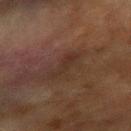Recorded during total-body skin imaging; not selected for excision or biopsy.
The lesion is located on the right forearm.
Approximately 4 mm at its widest.
The patient is a male aged approximately 70.
Automated image analysis of the tile measured a lesion area of about 5 mm². The software also gave a mean CIELAB color near L≈25 a*≈14 b*≈21 and a lesion-to-skin contrast of about 5.5 (normalized; higher = more distinct). The analysis additionally found a border-irregularity index near 5/10, internal color variation of about 1 on a 0–10 scale, and a peripheral color-asymmetry measure near 0.5. It also reported a classifier nevus-likeness of about 0/100 and a detector confidence of about 100 out of 100 that the crop contains a lesion.
A 15 mm close-up extracted from a 3D total-body photography capture.